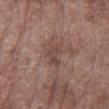Assessment:
Recorded during total-body skin imaging; not selected for excision or biopsy.
Context:
A roughly 15 mm field-of-view crop from a total-body skin photograph. A female subject, in their 70s. On the left forearm.